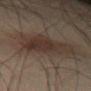Clinical impression: Captured during whole-body skin photography for melanoma surveillance; the lesion was not biopsied. Background: The total-body-photography lesion software estimated a border-irregularity index near 4.5/10. A male subject, aged 48 to 52. The lesion is located on the left leg. Captured under cross-polarized illumination. A 15 mm crop from a total-body photograph taken for skin-cancer surveillance.